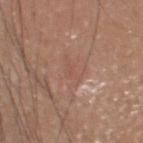subject — male, approximately 60 years of age
automated lesion analysis — an area of roughly 2 mm² and two-axis asymmetry of about 0.55; an average lesion color of about L≈52 a*≈22 b*≈27 (CIELAB), about 5 CIELAB-L* units darker than the surrounding skin, and a lesion-to-skin contrast of about 3.5 (normalized; higher = more distinct); a color-variation rating of about 0/10 and radial color variation of about 0; a classifier nevus-likeness of about 0/100 and a lesion-detection confidence of about 50/100
lesion diameter — ~2 mm (longest diameter)
image source — ~15 mm crop, total-body skin-cancer survey
tile lighting — white-light illumination
anatomic site — the head or neck